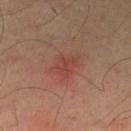Notes:
* biopsy status — no biopsy performed (imaged during a skin exam)
* acquisition — ~15 mm crop, total-body skin-cancer survey
* patient — male, about 55 years old
* body site — the left lower leg